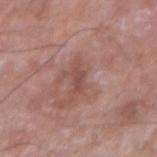image = total-body-photography crop, ~15 mm field of view; site = the right upper arm; lesion diameter = about 3 mm; patient = male, aged around 65.